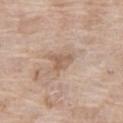workup=total-body-photography surveillance lesion; no biopsy
imaging modality=15 mm crop, total-body photography
lighting=white-light illumination
lesion diameter=~3 mm (longest diameter)
subject=female, roughly 75 years of age
site=the right thigh